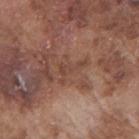Q: Is there a histopathology result?
A: imaged on a skin check; not biopsied
Q: How was the tile lit?
A: white-light
Q: Where on the body is the lesion?
A: the right upper arm
Q: Patient demographics?
A: male, aged 73 to 77
Q: How was this image acquired?
A: ~15 mm crop, total-body skin-cancer survey